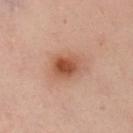follow-up = no biopsy performed (imaged during a skin exam) | imaging modality = ~15 mm tile from a whole-body skin photo | illumination = cross-polarized illumination | location = the chest | image-analysis metrics = a lesion color around L≈42 a*≈21 b*≈28 in CIELAB, roughly 11 lightness units darker than nearby skin, and a normalized border contrast of about 9; a border-irregularity index near 1.5/10, a within-lesion color-variation index near 4.5/10, and radial color variation of about 1.5 | lesion diameter = ~3.5 mm (longest diameter) | patient = female, in their mid- to late 50s.